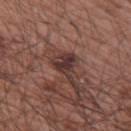The lesion was tiled from a total-body skin photograph and was not biopsied.
A male patient, aged approximately 65.
The lesion-visualizer software estimated an area of roughly 6 mm², an eccentricity of roughly 0.8, and a symmetry-axis asymmetry near 0.5. The analysis additionally found a border-irregularity index near 6/10 and radial color variation of about 1.
Cropped from a total-body skin-imaging series; the visible field is about 15 mm.
This is a white-light tile.
Longest diameter approximately 4 mm.
The lesion is located on the right upper arm.